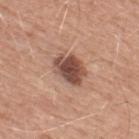{"biopsy_status": "not biopsied; imaged during a skin examination", "automated_metrics": {"cielab_L": 49, "cielab_a": 22, "cielab_b": 26, "vs_skin_darker_L": 15.0, "nevus_likeness_0_100": 50, "lesion_detection_confidence_0_100": 100}, "patient": {"sex": "male", "age_approx": 50}, "lighting": "white-light", "image": {"source": "total-body photography crop", "field_of_view_mm": 15}, "site": "upper back", "lesion_size": {"long_diameter_mm_approx": 4.5}}